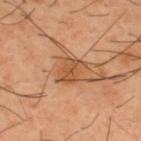follow-up: no biopsy performed (imaged during a skin exam)
imaging modality: ~15 mm crop, total-body skin-cancer survey
patient: male, aged 38 to 42
site: the upper back
diameter: about 3.5 mm
automated lesion analysis: a footprint of about 6 mm² and an eccentricity of roughly 0.7; a border-irregularity rating of about 3.5/10 and a color-variation rating of about 2.5/10